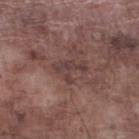No biopsy was performed on this lesion — it was imaged during a full skin examination and was not determined to be concerning. Longest diameter approximately 3.5 mm. The lesion is located on the left lower leg. A 15 mm crop from a total-body photograph taken for skin-cancer surveillance. Captured under white-light illumination. A male patient, approximately 75 years of age.